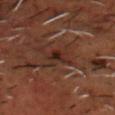Recorded during total-body skin imaging; not selected for excision or biopsy.
A male patient, aged approximately 50.
This is a cross-polarized tile.
A 15 mm close-up tile from a total-body photography series done for melanoma screening.
From the head or neck.
The total-body-photography lesion software estimated a lesion color around L≈21 a*≈16 b*≈19 in CIELAB, roughly 6 lightness units darker than nearby skin, and a normalized lesion–skin contrast near 7. It also reported a border-irregularity index near 3.5/10 and a within-lesion color-variation index near 6/10. The software also gave a classifier nevus-likeness of about 25/100 and lesion-presence confidence of about 75/100.